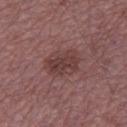Q: Was this lesion biopsied?
A: imaged on a skin check; not biopsied
Q: What are the patient's age and sex?
A: male, approximately 50 years of age
Q: What is the anatomic site?
A: the left lower leg
Q: What kind of image is this?
A: ~15 mm tile from a whole-body skin photo